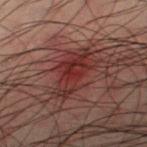| field | value |
|---|---|
| biopsy status | catalogued during a skin exam; not biopsied |
| automated metrics | an average lesion color of about L≈23 a*≈21 b*≈18 (CIELAB), about 6 CIELAB-L* units darker than the surrounding skin, and a normalized lesion–skin contrast near 7.5; an automated nevus-likeness rating near 25 out of 100 and a lesion-detection confidence of about 100/100 |
| illumination | cross-polarized illumination |
| patient | male, aged 48 to 52 |
| anatomic site | the leg |
| image | 15 mm crop, total-body photography |
| size | ≈6 mm |